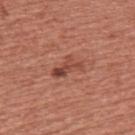<tbp_lesion>
  <biopsy_status>not biopsied; imaged during a skin examination</biopsy_status>
  <patient>
    <sex>male</sex>
    <age_approx>45</age_approx>
  </patient>
  <site>chest</site>
  <image>
    <source>total-body photography crop</source>
    <field_of_view_mm>15</field_of_view_mm>
  </image>
</tbp_lesion>